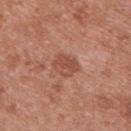Q: Was this lesion biopsied?
A: total-body-photography surveillance lesion; no biopsy
Q: Illumination type?
A: white-light
Q: What is the lesion's diameter?
A: ≈3 mm
Q: Patient demographics?
A: female, aged 38 to 42
Q: Where on the body is the lesion?
A: the back
Q: Automated lesion metrics?
A: an area of roughly 6 mm² and a shape eccentricity near 0.6; a lesion color around L≈50 a*≈25 b*≈29 in CIELAB, a lesion–skin lightness drop of about 9, and a lesion-to-skin contrast of about 6.5 (normalized; higher = more distinct); a nevus-likeness score of about 10/100 and a detector confidence of about 100 out of 100 that the crop contains a lesion
Q: What kind of image is this?
A: total-body-photography crop, ~15 mm field of view